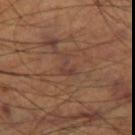{"biopsy_status": "not biopsied; imaged during a skin examination", "image": {"source": "total-body photography crop", "field_of_view_mm": 15}, "patient": {"sex": "male", "age_approx": 70}, "lighting": "cross-polarized", "lesion_size": {"long_diameter_mm_approx": 2.5}, "site": "right thigh"}